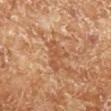Assessment: The lesion was photographed on a routine skin check and not biopsied; there is no pathology result. Image and clinical context: A male patient aged 68 to 72. Captured under cross-polarized illumination. The lesion's longest dimension is about 4 mm. Cropped from a total-body skin-imaging series; the visible field is about 15 mm. The lesion is located on the right lower leg.